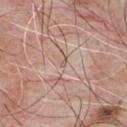Captured during whole-body skin photography for melanoma surveillance; the lesion was not biopsied. A male patient, aged 63 to 67. The recorded lesion diameter is about 1 mm. The lesion is on the front of the torso. The total-body-photography lesion software estimated an average lesion color of about L≈58 a*≈15 b*≈23 (CIELAB), about 8 CIELAB-L* units darker than the surrounding skin, and a normalized border contrast of about 5. And it measured a nevus-likeness score of about 0/100. This is a white-light tile. Cropped from a whole-body photographic skin survey; the tile spans about 15 mm.